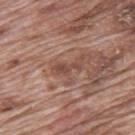Findings:
* site: the mid back
* subject: male, aged around 70
* size: ≈3 mm
* illumination: white-light
* image: 15 mm crop, total-body photography